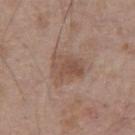{
  "biopsy_status": "not biopsied; imaged during a skin examination"
}